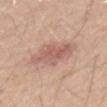The lesion was tiled from a total-body skin photograph and was not biopsied. The patient is a male about 65 years old. A region of skin cropped from a whole-body photographic capture, roughly 15 mm wide. Located on the mid back. Captured under white-light illumination.